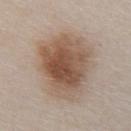Findings:
- notes: total-body-photography surveillance lesion; no biopsy
- subject: male, aged around 80
- imaging modality: 15 mm crop, total-body photography
- illumination: white-light
- TBP lesion metrics: an area of roughly 37 mm²
- site: the abdomen
- diameter: ~8 mm (longest diameter)